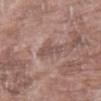No biopsy was performed on this lesion — it was imaged during a full skin examination and was not determined to be concerning. This is a white-light tile. The lesion is on the left forearm. Longest diameter approximately 3 mm. Automated tile analysis of the lesion measured a footprint of about 4 mm², an eccentricity of roughly 0.85, and a shape-asymmetry score of about 0.3 (0 = symmetric). A 15 mm close-up extracted from a 3D total-body photography capture. The patient is a female aged 68 to 72.